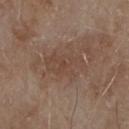This lesion was catalogued during total-body skin photography and was not selected for biopsy. The lesion is on the chest. Captured under white-light illumination. The total-body-photography lesion software estimated a shape eccentricity near 0.85 and a shape-asymmetry score of about 0.3 (0 = symmetric). It also reported internal color variation of about 2 on a 0–10 scale and peripheral color asymmetry of about 0.5. The software also gave an automated nevus-likeness rating near 0 out of 100 and a lesion-detection confidence of about 100/100. Cropped from a total-body skin-imaging series; the visible field is about 15 mm. A male patient aged 78–82. About 5 mm across.